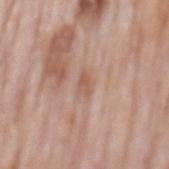follow-up — no biopsy performed (imaged during a skin exam)
automated metrics — an area of roughly 2.5 mm², a shape eccentricity near 0.85, and a symmetry-axis asymmetry near 0.35; a normalized border contrast of about 5.5
image — total-body-photography crop, ~15 mm field of view
anatomic site — the mid back
subject — male, aged 73 to 77
lesion size — ~2.5 mm (longest diameter)